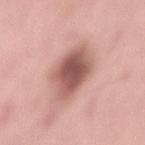site: lower back
lesion_size:
  long_diameter_mm_approx: 4.5
lighting: white-light
image:
  source: total-body photography crop
  field_of_view_mm: 15
automated_metrics:
  nevus_likeness_0_100: 55
  lesion_detection_confidence_0_100: 100
patient:
  sex: male
  age_approx: 55